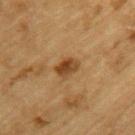The lesion was photographed on a routine skin check and not biopsied; there is no pathology result.
A male patient aged 83 or over.
Approximately 3 mm at its widest.
A roughly 15 mm field-of-view crop from a total-body skin photograph.
The tile uses cross-polarized illumination.
The lesion is located on the left upper arm.
An algorithmic analysis of the crop reported a border-irregularity rating of about 2/10, internal color variation of about 4 on a 0–10 scale, and peripheral color asymmetry of about 1. The software also gave a lesion-detection confidence of about 100/100.